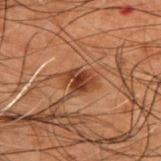No biopsy was performed on this lesion — it was imaged during a full skin examination and was not determined to be concerning. A male subject, about 50 years old. Located on the upper back. A 15 mm close-up tile from a total-body photography series done for melanoma screening.